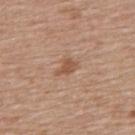| key | value |
|---|---|
| workup | no biopsy performed (imaged during a skin exam) |
| subject | female, aged 58–62 |
| location | the upper back |
| acquisition | 15 mm crop, total-body photography |
| illumination | white-light illumination |